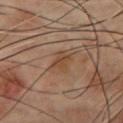illumination=cross-polarized
automated metrics=an average lesion color of about L≈42 a*≈18 b*≈30 (CIELAB) and a normalized lesion–skin contrast near 6.5; a border-irregularity index near 3/10 and peripheral color asymmetry of about 1; a nevus-likeness score of about 0/100 and a lesion-detection confidence of about 100/100
location=the front of the torso
subject=male, in their 70s
image source=~15 mm crop, total-body skin-cancer survey